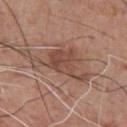Captured during whole-body skin photography for melanoma surveillance; the lesion was not biopsied.
A male subject about 60 years old.
Automated image analysis of the tile measured an average lesion color of about L≈48 a*≈20 b*≈26 (CIELAB), roughly 9 lightness units darker than nearby skin, and a lesion-to-skin contrast of about 7 (normalized; higher = more distinct). The analysis additionally found border irregularity of about 8 on a 0–10 scale, a color-variation rating of about 4/10, and radial color variation of about 1.5.
From the front of the torso.
A 15 mm close-up tile from a total-body photography series done for melanoma screening.